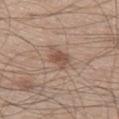The lesion was tiled from a total-body skin photograph and was not biopsied.